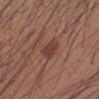workup — imaged on a skin check; not biopsied
site — the left forearm
patient — male, roughly 25 years of age
lesion diameter — about 3 mm
imaging modality — ~15 mm tile from a whole-body skin photo
image-analysis metrics — a lesion-to-skin contrast of about 7 (normalized; higher = more distinct); a border-irregularity index near 2.5/10 and peripheral color asymmetry of about 0.5; a nevus-likeness score of about 60/100
lighting — white-light illumination